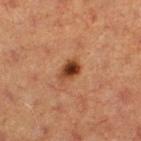{"biopsy_status": "not biopsied; imaged during a skin examination", "site": "leg", "automated_metrics": {"area_mm2_approx": 4.5, "eccentricity": 0.7, "shape_asymmetry": 0.25, "vs_skin_darker_L": 13.0, "vs_skin_contrast_norm": 11.5, "border_irregularity_0_10": 2.5, "color_variation_0_10": 5.5, "peripheral_color_asymmetry": 2.0, "lesion_detection_confidence_0_100": 100}, "image": {"source": "total-body photography crop", "field_of_view_mm": 15}, "lesion_size": {"long_diameter_mm_approx": 3.0}, "patient": {"sex": "female", "age_approx": 40}, "lighting": "cross-polarized"}